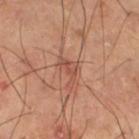Recorded during total-body skin imaging; not selected for excision or biopsy. The patient is a male aged 58 to 62. The lesion is located on the right thigh. Cropped from a whole-body photographic skin survey; the tile spans about 15 mm. Measured at roughly 3.5 mm in maximum diameter. This is a cross-polarized tile.